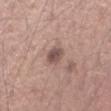Captured during whole-body skin photography for melanoma surveillance; the lesion was not biopsied.
Located on the left forearm.
The subject is a male aged around 45.
Imaged with white-light lighting.
A 15 mm crop from a total-body photograph taken for skin-cancer surveillance.